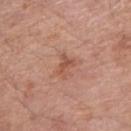Captured during whole-body skin photography for melanoma surveillance; the lesion was not biopsied.
Captured under white-light illumination.
Longest diameter approximately 3 mm.
The lesion is located on the right upper arm.
A roughly 15 mm field-of-view crop from a total-body skin photograph.
A male subject, aged 68 to 72.